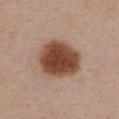The lesion was tiled from a total-body skin photograph and was not biopsied.
Imaged with white-light lighting.
A 15 mm crop from a total-body photograph taken for skin-cancer surveillance.
The patient is a female roughly 55 years of age.
The lesion is located on the chest.
Longest diameter approximately 6 mm.